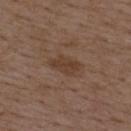• notes: total-body-photography surveillance lesion; no biopsy
• anatomic site: the upper back
• image: ~15 mm crop, total-body skin-cancer survey
• illumination: white-light illumination
• image-analysis metrics: a mean CIELAB color near L≈39 a*≈17 b*≈27, about 7 CIELAB-L* units darker than the surrounding skin, and a normalized border contrast of about 6.5; an automated nevus-likeness rating near 5 out of 100
• patient: male, in their 50s
• lesion size: about 4 mm